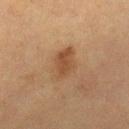Clinical impression: Imaged during a routine full-body skin examination; the lesion was not biopsied and no histopathology is available. Clinical summary: Located on the right lower leg. Cropped from a total-body skin-imaging series; the visible field is about 15 mm. Longest diameter approximately 3.5 mm. A female patient aged approximately 55. Automated image analysis of the tile measured an area of roughly 9 mm², an eccentricity of roughly 0.6, and a shape-asymmetry score of about 0.15 (0 = symmetric). It also reported an average lesion color of about L≈40 a*≈17 b*≈29 (CIELAB), about 8 CIELAB-L* units darker than the surrounding skin, and a lesion-to-skin contrast of about 7 (normalized; higher = more distinct). And it measured a within-lesion color-variation index near 3/10 and radial color variation of about 1.